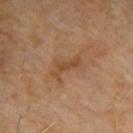Assessment:
The lesion was photographed on a routine skin check and not biopsied; there is no pathology result.
Acquisition and patient details:
A male patient roughly 65 years of age. A 15 mm close-up tile from a total-body photography series done for melanoma screening. Located on the right upper arm. An algorithmic analysis of the crop reported a shape eccentricity near 0.85 and a shape-asymmetry score of about 0.65 (0 = symmetric). It also reported a border-irregularity rating of about 8/10, internal color variation of about 0.5 on a 0–10 scale, and radial color variation of about 0. The software also gave a lesion-detection confidence of about 100/100. This is a cross-polarized tile. Longest diameter approximately 4 mm.